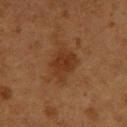Findings:
– site · the back
– imaging modality · ~15 mm crop, total-body skin-cancer survey
– image-analysis metrics · a mean CIELAB color near L≈30 a*≈20 b*≈29, about 7 CIELAB-L* units darker than the surrounding skin, and a normalized border contrast of about 7
– size · about 3.5 mm
– patient · male, aged 53 to 57